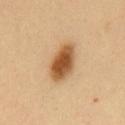Impression:
Part of a total-body skin-imaging series; this lesion was reviewed on a skin check and was not flagged for biopsy.
Context:
Captured under cross-polarized illumination. A region of skin cropped from a whole-body photographic capture, roughly 15 mm wide. A male patient approximately 40 years of age. From the chest. Longest diameter approximately 5 mm.